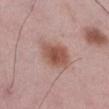No biopsy was performed on this lesion — it was imaged during a full skin examination and was not determined to be concerning. An algorithmic analysis of the crop reported an eccentricity of roughly 0.5 and two-axis asymmetry of about 0.15. The lesion's longest dimension is about 5.5 mm. A male patient aged 48–52. This image is a 15 mm lesion crop taken from a total-body photograph. The lesion is on the left lower leg. Imaged with white-light lighting.